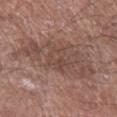Assessment: Part of a total-body skin-imaging series; this lesion was reviewed on a skin check and was not flagged for biopsy. Background: Longest diameter approximately 9 mm. Captured under white-light illumination. Cropped from a total-body skin-imaging series; the visible field is about 15 mm. A male patient in their mid-70s. On the left forearm. The total-body-photography lesion software estimated an area of roughly 30 mm², a shape eccentricity near 0.85, and two-axis asymmetry of about 0.3. The software also gave a mean CIELAB color near L≈47 a*≈18 b*≈23 and a lesion-to-skin contrast of about 6 (normalized; higher = more distinct). The analysis additionally found border irregularity of about 5.5 on a 0–10 scale, internal color variation of about 4 on a 0–10 scale, and radial color variation of about 1.5. It also reported an automated nevus-likeness rating near 0 out of 100 and a detector confidence of about 80 out of 100 that the crop contains a lesion.